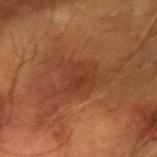Q: Was this lesion biopsied?
A: total-body-photography surveillance lesion; no biopsy
Q: What is the anatomic site?
A: the left forearm
Q: How large is the lesion?
A: ~5.5 mm (longest diameter)
Q: What lighting was used for the tile?
A: cross-polarized illumination
Q: What kind of image is this?
A: ~15 mm crop, total-body skin-cancer survey
Q: Patient demographics?
A: male, approximately 65 years of age